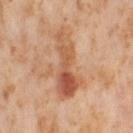Imaged during a routine full-body skin examination; the lesion was not biopsied and no histopathology is available.
A lesion tile, about 15 mm wide, cut from a 3D total-body photograph.
The lesion-visualizer software estimated an area of roughly 16 mm² and a shape eccentricity near 0.95. It also reported an average lesion color of about L≈57 a*≈24 b*≈35 (CIELAB), a lesion–skin lightness drop of about 11, and a normalized lesion–skin contrast near 7.5. It also reported an automated nevus-likeness rating near 5 out of 100 and a lesion-detection confidence of about 100/100.
The lesion's longest dimension is about 7.5 mm.
Captured under cross-polarized illumination.
On the right thigh.
A female patient aged 53 to 57.